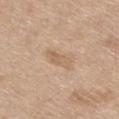Assessment: The lesion was photographed on a routine skin check and not biopsied; there is no pathology result.